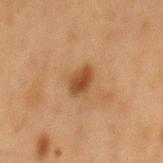Findings:
- biopsy status · total-body-photography surveillance lesion; no biopsy
- image source · ~15 mm crop, total-body skin-cancer survey
- size · about 3 mm
- body site · the mid back
- patient · male, in their mid- to late 70s